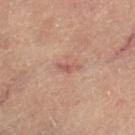{"biopsy_status": "not biopsied; imaged during a skin examination", "lesion_size": {"long_diameter_mm_approx": 3.0}, "site": "left thigh", "lighting": "cross-polarized", "image": {"source": "total-body photography crop", "field_of_view_mm": 15}, "patient": {"sex": "female", "age_approx": 65}}